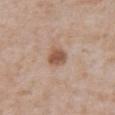<lesion>
<biopsy_status>not biopsied; imaged during a skin examination</biopsy_status>
<lesion_size>
  <long_diameter_mm_approx>2.5</long_diameter_mm_approx>
</lesion_size>
<site>abdomen</site>
<patient>
  <sex>male</sex>
  <age_approx>65</age_approx>
</patient>
<automated_metrics>
  <nevus_likeness_0_100>90</nevus_likeness_0_100>
  <lesion_detection_confidence_0_100>100</lesion_detection_confidence_0_100>
</automated_metrics>
<image>
  <source>total-body photography crop</source>
  <field_of_view_mm>15</field_of_view_mm>
</image>
<lighting>white-light</lighting>
</lesion>